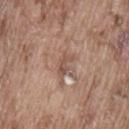Measured at roughly 3 mm in maximum diameter.
A close-up tile cropped from a whole-body skin photograph, about 15 mm across.
Captured under white-light illumination.
The lesion is located on the lower back.
A male subject aged approximately 75.